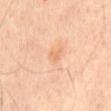biopsy_status: not biopsied; imaged during a skin examination
image:
  source: total-body photography crop
  field_of_view_mm: 15
automated_metrics:
  eccentricity: 0.55
  shape_asymmetry: 0.4
  border_irregularity_0_10: 3.0
site: front of the torso
patient:
  sex: male
  age_approx: 65